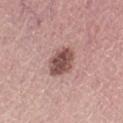Captured during whole-body skin photography for melanoma surveillance; the lesion was not biopsied.
The lesion is located on the left thigh.
This is a white-light tile.
A region of skin cropped from a whole-body photographic capture, roughly 15 mm wide.
A female subject, approximately 65 years of age.